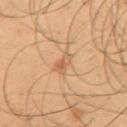{
  "biopsy_status": "not biopsied; imaged during a skin examination",
  "lighting": "cross-polarized",
  "patient": {
    "sex": "male",
    "age_approx": 55
  },
  "image": {
    "source": "total-body photography crop",
    "field_of_view_mm": 15
  },
  "lesion_size": {
    "long_diameter_mm_approx": 2.5
  },
  "site": "right upper arm"
}